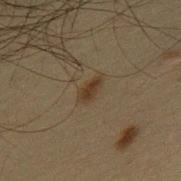  biopsy_status: not biopsied; imaged during a skin examination
  lighting: cross-polarized
  lesion_size:
    long_diameter_mm_approx: 3.0
  image:
    source: total-body photography crop
    field_of_view_mm: 15
  patient:
    sex: male
    age_approx: 65
  site: mid back
  automated_metrics:
    eccentricity: 0.9
    shape_asymmetry: 0.35
    nevus_likeness_0_100: 95
    lesion_detection_confidence_0_100: 100